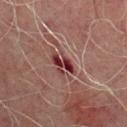workup: no biopsy performed (imaged during a skin exam) | imaging modality: 15 mm crop, total-body photography | subject: male, approximately 70 years of age | body site: the back.